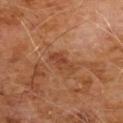Assessment:
The lesion was tiled from a total-body skin photograph and was not biopsied.
Context:
On the chest. Automated image analysis of the tile measured a border-irregularity index near 4.5/10, internal color variation of about 2 on a 0–10 scale, and peripheral color asymmetry of about 0.5. And it measured a classifier nevus-likeness of about 0/100 and a detector confidence of about 100 out of 100 that the crop contains a lesion. A region of skin cropped from a whole-body photographic capture, roughly 15 mm wide. A male subject aged 58–62.